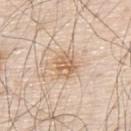* workup: catalogued during a skin exam; not biopsied
* image: total-body-photography crop, ~15 mm field of view
* patient: male, in their 80s
* TBP lesion metrics: border irregularity of about 2 on a 0–10 scale and internal color variation of about 4.5 on a 0–10 scale
* site: the upper back
* illumination: white-light illumination
* lesion diameter: about 3 mm